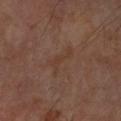Recorded during total-body skin imaging; not selected for excision or biopsy.
The lesion is located on the right forearm.
Cropped from a whole-body photographic skin survey; the tile spans about 15 mm.
The patient is a male approximately 70 years of age.
The total-body-photography lesion software estimated roughly 4 lightness units darker than nearby skin. The analysis additionally found border irregularity of about 7.5 on a 0–10 scale, internal color variation of about 0 on a 0–10 scale, and a peripheral color-asymmetry measure near 0.
Captured under cross-polarized illumination.
Approximately 4 mm at its widest.